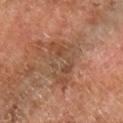biopsy status — no biopsy performed (imaged during a skin exam)